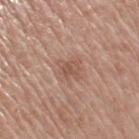Assessment:
Recorded during total-body skin imaging; not selected for excision or biopsy.
Image and clinical context:
A close-up tile cropped from a whole-body skin photograph, about 15 mm across. The lesion is located on the arm. A female patient aged approximately 75.